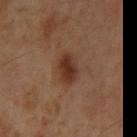workup: total-body-photography surveillance lesion; no biopsy | lighting: cross-polarized | imaging modality: total-body-photography crop, ~15 mm field of view | site: the back | automated lesion analysis: a lesion color around L≈30 a*≈19 b*≈26 in CIELAB, roughly 9 lightness units darker than nearby skin, and a lesion-to-skin contrast of about 9 (normalized; higher = more distinct); a nevus-likeness score of about 90/100 and a detector confidence of about 100 out of 100 that the crop contains a lesion | diameter: about 4 mm | subject: male, aged 58 to 62.